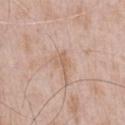Assessment: The lesion was photographed on a routine skin check and not biopsied; there is no pathology result. Image and clinical context: Cropped from a whole-body photographic skin survey; the tile spans about 15 mm. A male subject in their 50s. The lesion is on the front of the torso. Automated image analysis of the tile measured two-axis asymmetry of about 0.35. The software also gave a lesion color around L≈62 a*≈18 b*≈30 in CIELAB, roughly 7 lightness units darker than nearby skin, and a normalized lesion–skin contrast near 5.5. The software also gave a border-irregularity index near 3.5/10, internal color variation of about 1.5 on a 0–10 scale, and peripheral color asymmetry of about 0.5. The analysis additionally found lesion-presence confidence of about 100/100.